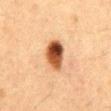This lesion was catalogued during total-body skin photography and was not selected for biopsy.
A lesion tile, about 15 mm wide, cut from a 3D total-body photograph.
The lesion is on the front of the torso.
A male subject roughly 50 years of age.